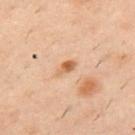biopsy status: no biopsy performed (imaged during a skin exam)
acquisition: 15 mm crop, total-body photography
subject: male, in their 40s
TBP lesion metrics: a lesion area of about 2.5 mm², a shape eccentricity near 0.85, and two-axis asymmetry of about 0.3; a lesion color around L≈60 a*≈23 b*≈38 in CIELAB, about 12 CIELAB-L* units darker than the surrounding skin, and a normalized lesion–skin contrast near 9; a border-irregularity index near 3/10, a within-lesion color-variation index near 2/10, and peripheral color asymmetry of about 0.5; a nevus-likeness score of about 5/100
site: the upper back